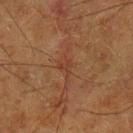Context: Located on the left lower leg. The lesion-visualizer software estimated an outline eccentricity of about 0.95 (0 = round, 1 = elongated) and a shape-asymmetry score of about 0.5 (0 = symmetric). The software also gave roughly 5 lightness units darker than nearby skin and a normalized border contrast of about 5. The software also gave a border-irregularity rating of about 7.5/10 and radial color variation of about 0.5. A male subject, aged 63–67. The tile uses cross-polarized illumination. The recorded lesion diameter is about 5 mm. A roughly 15 mm field-of-view crop from a total-body skin photograph.